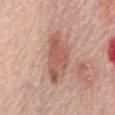Q: Was a biopsy performed?
A: total-body-photography surveillance lesion; no biopsy
Q: What is the anatomic site?
A: the front of the torso
Q: What did automated image analysis measure?
A: an area of roughly 16 mm² and a shape-asymmetry score of about 0.25 (0 = symmetric); border irregularity of about 3 on a 0–10 scale, internal color variation of about 4 on a 0–10 scale, and radial color variation of about 1.5; a classifier nevus-likeness of about 35/100
Q: What is the imaging modality?
A: ~15 mm tile from a whole-body skin photo
Q: Patient demographics?
A: female, aged 63 to 67
Q: How large is the lesion?
A: ≈7 mm